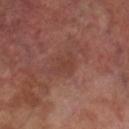No biopsy was performed on this lesion — it was imaged during a full skin examination and was not determined to be concerning. A male patient, in their 70s. Located on the right lower leg. Captured under cross-polarized illumination. A lesion tile, about 15 mm wide, cut from a 3D total-body photograph. About 3 mm across. The lesion-visualizer software estimated an average lesion color of about L≈38 a*≈22 b*≈25 (CIELAB), roughly 5 lightness units darker than nearby skin, and a normalized border contrast of about 4.5. And it measured an automated nevus-likeness rating near 0 out of 100.